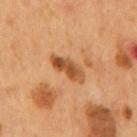Acquisition and patient details:
Cropped from a whole-body photographic skin survey; the tile spans about 15 mm. A male subject, aged 53 to 57. The lesion is located on the back.